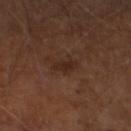Impression:
The lesion was photographed on a routine skin check and not biopsied; there is no pathology result.
Background:
On the leg. The subject is a male in their 60s. The recorded lesion diameter is about 3.5 mm. A close-up tile cropped from a whole-body skin photograph, about 15 mm across.